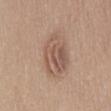  biopsy_status: not biopsied; imaged during a skin examination
  lighting: white-light
  lesion_size:
    long_diameter_mm_approx: 5.5
  site: abdomen
  automated_metrics:
    area_mm2_approx: 14.0
    eccentricity: 0.7
    shape_asymmetry: 0.3
    vs_skin_darker_L: 9.0
    vs_skin_contrast_norm: 6.5
    color_variation_0_10: 5.5
    nevus_likeness_0_100: 20
    lesion_detection_confidence_0_100: 100
  patient:
    sex: male
    age_approx: 50
  image:
    source: total-body photography crop
    field_of_view_mm: 15